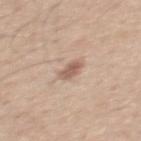Captured during whole-body skin photography for melanoma surveillance; the lesion was not biopsied. Located on the back. Cropped from a total-body skin-imaging series; the visible field is about 15 mm. Longest diameter approximately 3 mm. Imaged with white-light lighting. The patient is a male aged around 55.